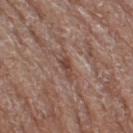This lesion was catalogued during total-body skin photography and was not selected for biopsy. Measured at roughly 3 mm in maximum diameter. A 15 mm close-up extracted from a 3D total-body photography capture. An algorithmic analysis of the crop reported an area of roughly 2.5 mm² and two-axis asymmetry of about 0.35. The software also gave an average lesion color of about L≈44 a*≈20 b*≈24 (CIELAB) and a lesion–skin lightness drop of about 9. The analysis additionally found border irregularity of about 4 on a 0–10 scale and radial color variation of about 0. A female subject, approximately 75 years of age. Located on the right thigh.